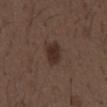{
  "biopsy_status": "not biopsied; imaged during a skin examination"
}